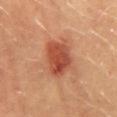The lesion was tiled from a total-body skin photograph and was not biopsied. Measured at roughly 4.5 mm in maximum diameter. The lesion is on the back. A female patient, in their 30s. A 15 mm crop from a total-body photograph taken for skin-cancer surveillance.